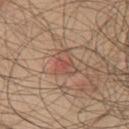Q: Was this lesion biopsied?
A: no biopsy performed (imaged during a skin exam)
Q: How was this image acquired?
A: total-body-photography crop, ~15 mm field of view
Q: What is the anatomic site?
A: the chest
Q: What lighting was used for the tile?
A: white-light illumination
Q: What is the lesion's diameter?
A: about 3.5 mm
Q: What did automated image analysis measure?
A: a lesion color around L≈52 a*≈22 b*≈28 in CIELAB and roughly 7 lightness units darker than nearby skin; a detector confidence of about 100 out of 100 that the crop contains a lesion
Q: Patient demographics?
A: male, approximately 45 years of age